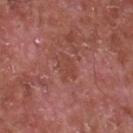This lesion was catalogued during total-body skin photography and was not selected for biopsy.
An algorithmic analysis of the crop reported a mean CIELAB color near L≈45 a*≈26 b*≈27, a lesion–skin lightness drop of about 5, and a lesion-to-skin contrast of about 4.5 (normalized; higher = more distinct). And it measured an automated nevus-likeness rating near 0 out of 100 and a lesion-detection confidence of about 100/100.
Longest diameter approximately 3 mm.
From the chest.
A male subject aged 63 to 67.
A 15 mm crop from a total-body photograph taken for skin-cancer surveillance.